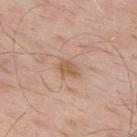The subject is a male aged 63–67. Approximately 2.5 mm at its widest. The tile uses white-light illumination. Located on the upper back. A roughly 15 mm field-of-view crop from a total-body skin photograph.